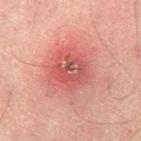biopsy_status: not biopsied; imaged during a skin examination
automated_metrics:
  border_irregularity_0_10: 2.5
  color_variation_0_10: 7.5
  peripheral_color_asymmetry: 3.0
lighting: cross-polarized
lesion_size:
  long_diameter_mm_approx: 4.0
patient:
  sex: male
  age_approx: 30
image:
  source: total-body photography crop
  field_of_view_mm: 15
site: leg